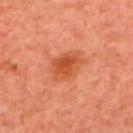workup: catalogued during a skin exam; not biopsied | lesion diameter: about 4.5 mm | location: the upper back | subject: male, about 65 years old | illumination: cross-polarized | image: total-body-photography crop, ~15 mm field of view.